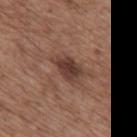A 15 mm close-up tile from a total-body photography series done for melanoma screening.
The lesion's longest dimension is about 4 mm.
A male patient, aged 68 to 72.
The lesion is on the upper back.
This is a white-light tile.